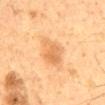image — total-body-photography crop, ~15 mm field of view
subject — male, aged around 60
location — the mid back
size — about 4.5 mm
tile lighting — cross-polarized
image-analysis metrics — a shape eccentricity near 0.85 and two-axis asymmetry of about 0.25; an average lesion color of about L≈57 a*≈20 b*≈38 (CIELAB), roughly 9 lightness units darker than nearby skin, and a normalized border contrast of about 6; lesion-presence confidence of about 100/100